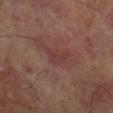{
  "patient": {
    "sex": "male",
    "age_approx": 70
  },
  "lesion_size": {
    "long_diameter_mm_approx": 3.0
  },
  "image": {
    "source": "total-body photography crop",
    "field_of_view_mm": 15
  },
  "lighting": "cross-polarized",
  "site": "leg"
}